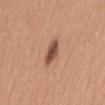workup — total-body-photography surveillance lesion; no biopsy | lighting — white-light | anatomic site — the back | image — 15 mm crop, total-body photography | subject — female, in their 40s.